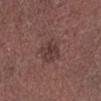This lesion was catalogued during total-body skin photography and was not selected for biopsy. A close-up tile cropped from a whole-body skin photograph, about 15 mm across. A male patient, aged approximately 65. From the left lower leg.